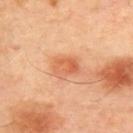| field | value |
|---|---|
| size | about 3.5 mm |
| site | the back |
| image-analysis metrics | an area of roughly 6.5 mm² and a shape-asymmetry score of about 0.25 (0 = symmetric); an average lesion color of about L≈65 a*≈29 b*≈40 (CIELAB) and roughly 10 lightness units darker than nearby skin; a border-irregularity index near 2.5/10; a nevus-likeness score of about 95/100 and lesion-presence confidence of about 100/100 |
| subject | female, aged approximately 40 |
| imaging modality | ~15 mm crop, total-body skin-cancer survey |
| tile lighting | cross-polarized illumination |